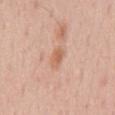| field | value |
|---|---|
| biopsy status | total-body-photography surveillance lesion; no biopsy |
| image | 15 mm crop, total-body photography |
| subject | male, roughly 55 years of age |
| illumination | white-light |
| automated lesion analysis | a footprint of about 4 mm², an eccentricity of roughly 0.8, and two-axis asymmetry of about 0.2; roughly 9 lightness units darker than nearby skin and a normalized lesion–skin contrast near 6.5; border irregularity of about 2 on a 0–10 scale, internal color variation of about 3 on a 0–10 scale, and a peripheral color-asymmetry measure near 1 |
| location | the mid back |
| diameter | about 3 mm |